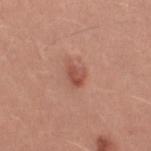follow-up: no biopsy performed (imaged during a skin exam)
illumination: white-light
image: 15 mm crop, total-body photography
patient: female, in their mid- to late 30s
diameter: about 2.5 mm
site: the left upper arm